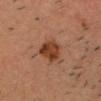Part of a total-body skin-imaging series; this lesion was reviewed on a skin check and was not flagged for biopsy.
A 15 mm close-up tile from a total-body photography series done for melanoma screening.
The tile uses cross-polarized illumination.
About 3.5 mm across.
On the head or neck.
A male patient, approximately 35 years of age.